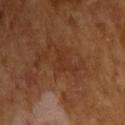| field | value |
|---|---|
| follow-up | total-body-photography surveillance lesion; no biopsy |
| tile lighting | cross-polarized |
| subject | male, approximately 65 years of age |
| image | ~15 mm crop, total-body skin-cancer survey |